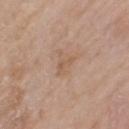Case summary:
- workup: no biopsy performed (imaged during a skin exam)
- acquisition: total-body-photography crop, ~15 mm field of view
- subject: female, aged around 85 or older
- size: ≈3 mm
- tile lighting: white-light illumination
- body site: the left upper arm